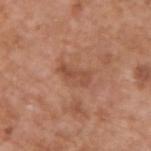workup: catalogued during a skin exam; not biopsied | image: 15 mm crop, total-body photography | lesion diameter: ≈4 mm | subject: male, aged approximately 65 | anatomic site: the upper back | tile lighting: white-light illumination.